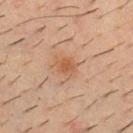The subject is a male aged 33–37. The lesion is located on the chest. Cropped from a total-body skin-imaging series; the visible field is about 15 mm.